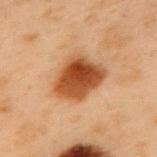The lesion was photographed on a routine skin check and not biopsied; there is no pathology result. A lesion tile, about 15 mm wide, cut from a 3D total-body photograph. The tile uses cross-polarized illumination. Longest diameter approximately 5 mm. A male patient aged 53 to 57. The lesion is located on the mid back.